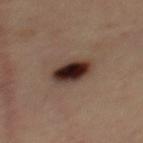Imaged during a routine full-body skin examination; the lesion was not biopsied and no histopathology is available. Automated tile analysis of the lesion measured an area of roughly 10 mm², a shape eccentricity near 0.7, and a shape-asymmetry score of about 0.15 (0 = symmetric). And it measured a classifier nevus-likeness of about 100/100 and lesion-presence confidence of about 100/100. Captured under cross-polarized illumination. The subject is a female about 45 years old. The lesion is located on the mid back. Longest diameter approximately 4 mm. A close-up tile cropped from a whole-body skin photograph, about 15 mm across.